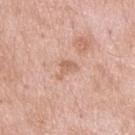  site: upper back
  lighting: white-light
  lesion_size:
    long_diameter_mm_approx: 3.0
  image:
    source: total-body photography crop
    field_of_view_mm: 15
  patient:
    sex: male
    age_approx: 55